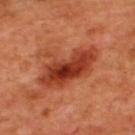image:
  source: total-body photography crop
  field_of_view_mm: 15
site: upper back
lesion_size:
  long_diameter_mm_approx: 6.5
lighting: cross-polarized
automated_metrics:
  eccentricity: 0.8
  shape_asymmetry: 0.35
  vs_skin_darker_L: 13.0
  vs_skin_contrast_norm: 9.5
  nevus_likeness_0_100: 60
  lesion_detection_confidence_0_100: 100
patient:
  sex: male
  age_approx: 50